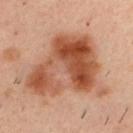| field | value |
|---|---|
| follow-up | total-body-photography surveillance lesion; no biopsy |
| illumination | cross-polarized illumination |
| image source | ~15 mm crop, total-body skin-cancer survey |
| anatomic site | the back |
| size | about 8.5 mm |
| subject | male, aged 38–42 |
| TBP lesion metrics | an area of roughly 37 mm², an outline eccentricity of about 0.65 (0 = round, 1 = elongated), and two-axis asymmetry of about 0.55; a lesion color around L≈50 a*≈25 b*≈34 in CIELAB, a lesion–skin lightness drop of about 14, and a lesion-to-skin contrast of about 10 (normalized; higher = more distinct); border irregularity of about 7.5 on a 0–10 scale and a within-lesion color-variation index near 7/10; a classifier nevus-likeness of about 25/100 |